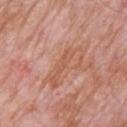  biopsy_status: not biopsied; imaged during a skin examination
  patient:
    sex: male
    age_approx: 80
  site: chest
  lighting: white-light
  image:
    source: total-body photography crop
    field_of_view_mm: 15